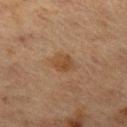biopsy_status: not biopsied; imaged during a skin examination
patient:
  sex: female
  age_approx: 40
site: left thigh
image:
  source: total-body photography crop
  field_of_view_mm: 15
lighting: cross-polarized